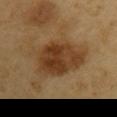Findings:
• notes · no biopsy performed (imaged during a skin exam)
• subject · male, aged approximately 60
• imaging modality · 15 mm crop, total-body photography
• anatomic site · the right upper arm